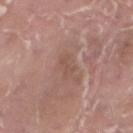Q: Is there a histopathology result?
A: total-body-photography surveillance lesion; no biopsy
Q: What is the anatomic site?
A: the right thigh
Q: What are the patient's age and sex?
A: male, about 40 years old
Q: Illumination type?
A: white-light
Q: What did automated image analysis measure?
A: border irregularity of about 4 on a 0–10 scale, a color-variation rating of about 0/10, and a peripheral color-asymmetry measure near 0; a detector confidence of about 95 out of 100 that the crop contains a lesion
Q: How was this image acquired?
A: 15 mm crop, total-body photography
Q: What is the lesion's diameter?
A: ≈2.5 mm